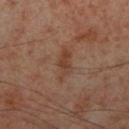Part of a total-body skin-imaging series; this lesion was reviewed on a skin check and was not flagged for biopsy. Imaged with cross-polarized lighting. About 4 mm across. A male patient about 55 years old. A 15 mm close-up tile from a total-body photography series done for melanoma screening. The lesion is on the right lower leg.